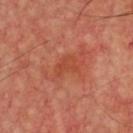The lesion was photographed on a routine skin check and not biopsied; there is no pathology result. Located on the chest. Cropped from a whole-body photographic skin survey; the tile spans about 15 mm. The subject is a male aged 48–52.